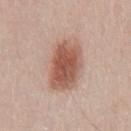{"biopsy_status": "not biopsied; imaged during a skin examination", "patient": {"sex": "male", "age_approx": 40}, "automated_metrics": {"eccentricity": 0.85, "shape_asymmetry": 0.2, "vs_skin_darker_L": 14.0, "vs_skin_contrast_norm": 9.5, "border_irregularity_0_10": 2.5, "color_variation_0_10": 5.0, "peripheral_color_asymmetry": 1.5, "nevus_likeness_0_100": 100}, "site": "mid back", "image": {"source": "total-body photography crop", "field_of_view_mm": 15}, "lighting": "white-light", "lesion_size": {"long_diameter_mm_approx": 7.0}}